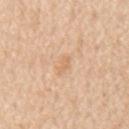Assessment:
Captured during whole-body skin photography for melanoma surveillance; the lesion was not biopsied.
Clinical summary:
Captured under white-light illumination. The lesion's longest dimension is about 2.5 mm. Automated image analysis of the tile measured a shape eccentricity near 0.9 and a shape-asymmetry score of about 0.4 (0 = symmetric). The analysis additionally found a mean CIELAB color near L≈68 a*≈19 b*≈37, roughly 7 lightness units darker than nearby skin, and a normalized border contrast of about 5. The analysis additionally found border irregularity of about 4 on a 0–10 scale and a peripheral color-asymmetry measure near 0. Located on the chest. A 15 mm close-up extracted from a 3D total-body photography capture. A male patient in their 70s.